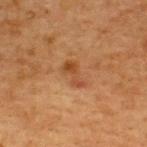No biopsy was performed on this lesion — it was imaged during a full skin examination and was not determined to be concerning.
Automated tile analysis of the lesion measured an area of roughly 5 mm² and an eccentricity of roughly 0.85. The analysis additionally found a classifier nevus-likeness of about 10/100 and a lesion-detection confidence of about 100/100.
On the back.
A female subject, in their 40s.
A 15 mm crop from a total-body photograph taken for skin-cancer surveillance.
This is a cross-polarized tile.
Longest diameter approximately 3.5 mm.